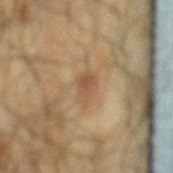Findings:
* biopsy status — total-body-photography surveillance lesion; no biopsy
* TBP lesion metrics — a lesion color around L≈49 a*≈16 b*≈31 in CIELAB, a lesion–skin lightness drop of about 8, and a lesion-to-skin contrast of about 6.5 (normalized; higher = more distinct); a border-irregularity index near 3/10, internal color variation of about 2.5 on a 0–10 scale, and radial color variation of about 1
* subject — male, in their mid-60s
* image source — ~15 mm crop, total-body skin-cancer survey
* body site — the mid back